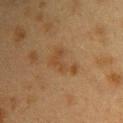biopsy status = no biopsy performed (imaged during a skin exam)
image source = total-body-photography crop, ~15 mm field of view
automated metrics = a footprint of about 6.5 mm² and an eccentricity of roughly 0.85
size = ~4 mm (longest diameter)
patient = female, roughly 40 years of age
location = the upper back